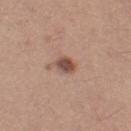biopsy status: catalogued during a skin exam; not biopsied
image: 15 mm crop, total-body photography
location: the left thigh
patient: female, roughly 45 years of age
diameter: ~2.5 mm (longest diameter)
tile lighting: white-light illumination
automated metrics: an area of roughly 4 mm², an eccentricity of roughly 0.7, and a shape-asymmetry score of about 0.15 (0 = symmetric); a border-irregularity index near 1.5/10, a within-lesion color-variation index near 3/10, and peripheral color asymmetry of about 1